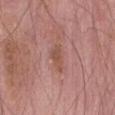{"site": "abdomen", "patient": {"sex": "male", "age_approx": 75}, "image": {"source": "total-body photography crop", "field_of_view_mm": 15}, "lesion_size": {"long_diameter_mm_approx": 3.5}}